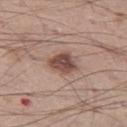– biopsy status: no biopsy performed (imaged during a skin exam)
– imaging modality: ~15 mm tile from a whole-body skin photo
– size: ≈3.5 mm
– location: the left thigh
– subject: male, aged 48 to 52
– automated lesion analysis: a mean CIELAB color near L≈47 a*≈19 b*≈24 and a normalized lesion–skin contrast near 10